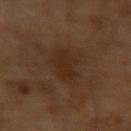Assessment:
Captured during whole-body skin photography for melanoma surveillance; the lesion was not biopsied.
Background:
A 15 mm close-up extracted from a 3D total-body photography capture. The lesion's longest dimension is about 4.5 mm. The tile uses cross-polarized illumination. A male patient, in their mid-60s. The lesion-visualizer software estimated a footprint of about 7 mm², an eccentricity of roughly 0.85, and a shape-asymmetry score of about 0.25 (0 = symmetric). And it measured roughly 5 lightness units darker than nearby skin and a normalized lesion–skin contrast near 6. The analysis additionally found a classifier nevus-likeness of about 0/100 and a detector confidence of about 100 out of 100 that the crop contains a lesion.